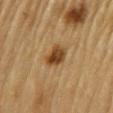Case summary:
– workup — no biopsy performed (imaged during a skin exam)
– automated lesion analysis — border irregularity of about 2 on a 0–10 scale, internal color variation of about 5 on a 0–10 scale, and peripheral color asymmetry of about 1.5
– patient — male, roughly 85 years of age or older
– tile lighting — cross-polarized illumination
– lesion diameter — ~3 mm (longest diameter)
– body site — the arm
– imaging modality — total-body-photography crop, ~15 mm field of view